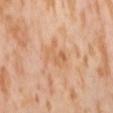Findings:
• follow-up — imaged on a skin check; not biopsied
• site — the lower back
• lesion diameter — ~4 mm (longest diameter)
• automated lesion analysis — a mean CIELAB color near L≈65 a*≈22 b*≈37 and a normalized border contrast of about 5; a border-irregularity index near 5.5/10, a color-variation rating of about 4.5/10, and peripheral color asymmetry of about 1.5
• image — ~15 mm crop, total-body skin-cancer survey
• patient — female, aged 53 to 57